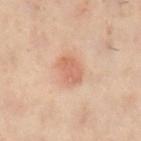Q: Was this lesion biopsied?
A: no biopsy performed (imaged during a skin exam)
Q: What are the patient's age and sex?
A: female, aged 58–62
Q: What is the anatomic site?
A: the left leg
Q: What is the imaging modality?
A: ~15 mm crop, total-body skin-cancer survey
Q: Automated lesion metrics?
A: an area of roughly 5.5 mm², an outline eccentricity of about 0.8 (0 = round, 1 = elongated), and a symmetry-axis asymmetry near 0.2; a classifier nevus-likeness of about 90/100 and a lesion-detection confidence of about 100/100
Q: Lesion size?
A: ~3.5 mm (longest diameter)
Q: What lighting was used for the tile?
A: cross-polarized illumination